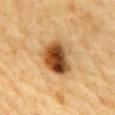Case summary:
• biopsy status — imaged on a skin check; not biopsied
• automated lesion analysis — a lesion area of about 15 mm², an outline eccentricity of about 0.65 (0 = round, 1 = elongated), and a shape-asymmetry score of about 0.2 (0 = symmetric); a border-irregularity rating of about 2/10, a color-variation rating of about 10/10, and a peripheral color-asymmetry measure near 3.5; an automated nevus-likeness rating near 100 out of 100 and a lesion-detection confidence of about 100/100
• image source — ~15 mm tile from a whole-body skin photo
• site — the abdomen
• tile lighting — cross-polarized illumination
• subject — male, roughly 85 years of age or older
• lesion size — ≈5 mm